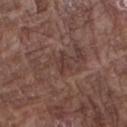Captured during whole-body skin photography for melanoma surveillance; the lesion was not biopsied. Automated image analysis of the tile measured a footprint of about 3.5 mm², a shape eccentricity near 0.7, and a shape-asymmetry score of about 0.35 (0 = symmetric). The software also gave roughly 6 lightness units darker than nearby skin. The software also gave a within-lesion color-variation index near 1.5/10 and radial color variation of about 0.5. A male patient aged 73 to 77. The lesion's longest dimension is about 2.5 mm. Located on the right forearm. A 15 mm close-up extracted from a 3D total-body photography capture.